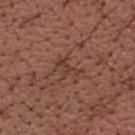Case summary:
– workup — catalogued during a skin exam; not biopsied
– lesion size — ~3 mm (longest diameter)
– subject — male, about 65 years old
– image — ~15 mm crop, total-body skin-cancer survey
– automated metrics — an eccentricity of roughly 0.85 and a shape-asymmetry score of about 0.5 (0 = symmetric); a color-variation rating of about 0/10 and peripheral color asymmetry of about 0; a nevus-likeness score of about 0/100 and lesion-presence confidence of about 70/100
– anatomic site — the head or neck